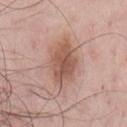The lesion was photographed on a routine skin check and not biopsied; there is no pathology result. The lesion is on the chest. A lesion tile, about 15 mm wide, cut from a 3D total-body photograph. Approximately 6 mm at its widest. The total-body-photography lesion software estimated an area of roughly 16 mm², an eccentricity of roughly 0.8, and a shape-asymmetry score of about 0.15 (0 = symmetric). And it measured a mean CIELAB color near L≈55 a*≈21 b*≈27, about 11 CIELAB-L* units darker than the surrounding skin, and a lesion-to-skin contrast of about 8 (normalized; higher = more distinct). It also reported a border-irregularity index near 2/10, a within-lesion color-variation index near 4.5/10, and peripheral color asymmetry of about 1.5. It also reported a nevus-likeness score of about 75/100 and lesion-presence confidence of about 100/100. A male patient, in their mid- to late 60s. Captured under white-light illumination.